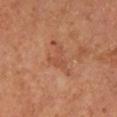Captured during whole-body skin photography for melanoma surveillance; the lesion was not biopsied. From the right lower leg. Cropped from a total-body skin-imaging series; the visible field is about 15 mm. Measured at roughly 4.5 mm in maximum diameter. Captured under cross-polarized illumination. The total-body-photography lesion software estimated a lesion area of about 6.5 mm² and two-axis asymmetry of about 0.65. The analysis additionally found roughly 7 lightness units darker than nearby skin and a lesion-to-skin contrast of about 5 (normalized; higher = more distinct). The analysis additionally found a border-irregularity index near 8.5/10 and a color-variation rating of about 2/10. And it measured an automated nevus-likeness rating near 0 out of 100 and lesion-presence confidence of about 100/100. A male subject, roughly 65 years of age.